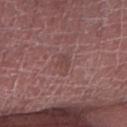{"biopsy_status": "not biopsied; imaged during a skin examination", "automated_metrics": {"vs_skin_darker_L": 6.0, "vs_skin_contrast_norm": 4.5, "border_irregularity_0_10": 3.0, "color_variation_0_10": 2.5, "peripheral_color_asymmetry": 1.0, "lesion_detection_confidence_0_100": 100}, "image": {"source": "total-body photography crop", "field_of_view_mm": 15}, "lighting": "white-light", "patient": {"sex": "male", "age_approx": 70}, "lesion_size": {"long_diameter_mm_approx": 3.0}, "site": "arm"}